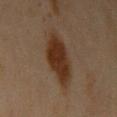{
  "biopsy_status": "not biopsied; imaged during a skin examination",
  "image": {
    "source": "total-body photography crop",
    "field_of_view_mm": 15
  },
  "site": "left upper arm",
  "patient": {
    "sex": "female",
    "age_approx": 45
  },
  "automated_metrics": {
    "border_irregularity_0_10": 2.0,
    "color_variation_0_10": 3.0,
    "peripheral_color_asymmetry": 1.0,
    "nevus_likeness_0_100": 100,
    "lesion_detection_confidence_0_100": 100
  },
  "lighting": "cross-polarized"
}